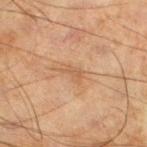Q: Was this lesion biopsied?
A: catalogued during a skin exam; not biopsied